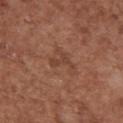  site: chest
  image:
    source: total-body photography crop
    field_of_view_mm: 15
  lighting: white-light
  patient:
    sex: female
    age_approx: 75
  automated_metrics:
    area_mm2_approx: 3.5
    eccentricity: 0.85
    shape_asymmetry: 0.6
    vs_skin_darker_L: 7.0
    vs_skin_contrast_norm: 5.5
    nevus_likeness_0_100: 0
    lesion_detection_confidence_0_100: 100
  lesion_size:
    long_diameter_mm_approx: 3.0